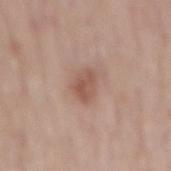biopsy status — catalogued during a skin exam; not biopsied
image source — 15 mm crop, total-body photography
site — the mid back
lesion diameter — about 3.5 mm
subject — male, aged around 80
illumination — white-light illumination